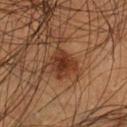<lesion>
  <image>
    <source>total-body photography crop</source>
    <field_of_view_mm>15</field_of_view_mm>
  </image>
  <site>left lower leg</site>
  <automated_metrics>
    <area_mm2_approx>7.5</area_mm2_approx>
    <eccentricity>0.65</eccentricity>
    <shape_asymmetry>0.35</shape_asymmetry>
    <cielab_L>33</cielab_L>
    <cielab_a>22</cielab_a>
    <cielab_b>30</cielab_b>
    <vs_skin_darker_L>10.0</vs_skin_darker_L>
    <vs_skin_contrast_norm>9.0</vs_skin_contrast_norm>
    <nevus_likeness_0_100>80</nevus_likeness_0_100>
    <lesion_detection_confidence_0_100>100</lesion_detection_confidence_0_100>
  </automated_metrics>
  <lighting>cross-polarized</lighting>
  <patient>
    <sex>male</sex>
    <age_approx>45</age_approx>
  </patient>
</lesion>